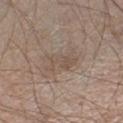* workup: imaged on a skin check; not biopsied
* patient: male, approximately 60 years of age
* TBP lesion metrics: an eccentricity of roughly 0.8; a lesion color around L≈51 a*≈13 b*≈24 in CIELAB, a lesion–skin lightness drop of about 6, and a lesion-to-skin contrast of about 5 (normalized; higher = more distinct); a nevus-likeness score of about 0/100 and a lesion-detection confidence of about 100/100
* body site: the right thigh
* acquisition: total-body-photography crop, ~15 mm field of view